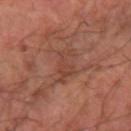A close-up tile cropped from a whole-body skin photograph, about 15 mm across. From the arm. A patient in their mid- to late 60s. The tile uses cross-polarized illumination.